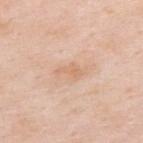The lesion was photographed on a routine skin check and not biopsied; there is no pathology result. The lesion is located on the upper back. A male subject, aged around 55. A roughly 15 mm field-of-view crop from a total-body skin photograph.